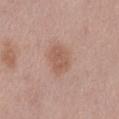No biopsy was performed on this lesion — it was imaged during a full skin examination and was not determined to be concerning.
Captured under white-light illumination.
The patient is a female aged approximately 40.
A 15 mm crop from a total-body photograph taken for skin-cancer surveillance.
Located on the left thigh.
Approximately 3 mm at its widest.